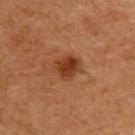Q: What are the patient's age and sex?
A: male, aged around 65
Q: What is the imaging modality?
A: 15 mm crop, total-body photography
Q: Illumination type?
A: cross-polarized
Q: Where on the body is the lesion?
A: the upper back